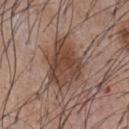follow-up: catalogued during a skin exam; not biopsied
site: the chest
patient: male, aged 53–57
image-analysis metrics: an average lesion color of about L≈43 a*≈19 b*≈26 (CIELAB), about 11 CIELAB-L* units darker than the surrounding skin, and a normalized border contrast of about 9; an automated nevus-likeness rating near 60 out of 100 and lesion-presence confidence of about 100/100
acquisition: ~15 mm crop, total-body skin-cancer survey
size: about 6 mm